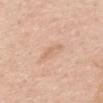| feature | finding |
|---|---|
| workup | catalogued during a skin exam; not biopsied |